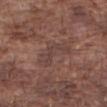{"biopsy_status": "not biopsied; imaged during a skin examination", "site": "right forearm", "image": {"source": "total-body photography crop", "field_of_view_mm": 15}, "automated_metrics": {"area_mm2_approx": 8.0, "eccentricity": 0.9, "shape_asymmetry": 0.5, "cielab_L": 41, "cielab_a": 18, "cielab_b": 21, "border_irregularity_0_10": 7.5, "color_variation_0_10": 1.5, "peripheral_color_asymmetry": 0.5}, "patient": {"sex": "male", "age_approx": 75}, "lesion_size": {"long_diameter_mm_approx": 4.5}, "lighting": "white-light"}